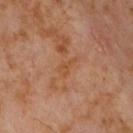Imaged during a routine full-body skin examination; the lesion was not biopsied and no histopathology is available.
From the chest.
Measured at roughly 2.5 mm in maximum diameter.
A male patient aged 58 to 62.
Automated tile analysis of the lesion measured a footprint of about 3 mm², a shape eccentricity near 0.85, and a symmetry-axis asymmetry near 0.5. The analysis additionally found a within-lesion color-variation index near 0.5/10 and peripheral color asymmetry of about 0.
Cropped from a total-body skin-imaging series; the visible field is about 15 mm.
Imaged with cross-polarized lighting.